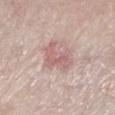No biopsy was performed on this lesion — it was imaged during a full skin examination and was not determined to be concerning. Cropped from a total-body skin-imaging series; the visible field is about 15 mm. The tile uses white-light illumination. From the left lower leg. The subject is a female approximately 65 years of age. The lesion-visualizer software estimated a footprint of about 9.5 mm², an eccentricity of roughly 0.8, and a shape-asymmetry score of about 0.35 (0 = symmetric). The analysis additionally found a lesion-to-skin contrast of about 6 (normalized; higher = more distinct). The software also gave a border-irregularity rating of about 5.5/10 and radial color variation of about 1.